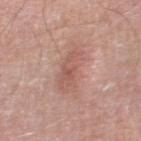follow-up = catalogued during a skin exam; not biopsied
automated metrics = a footprint of about 13 mm²; an average lesion color of about L≈57 a*≈22 b*≈26 (CIELAB) and a normalized lesion–skin contrast near 5.5; border irregularity of about 4.5 on a 0–10 scale, a within-lesion color-variation index near 3.5/10, and radial color variation of about 1
anatomic site = the right thigh
illumination = white-light
patient = male, roughly 80 years of age
lesion size = about 6.5 mm
acquisition = total-body-photography crop, ~15 mm field of view